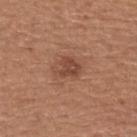<lesion>
<biopsy_status>not biopsied; imaged during a skin examination</biopsy_status>
<image>
  <source>total-body photography crop</source>
  <field_of_view_mm>15</field_of_view_mm>
</image>
<patient>
  <sex>female</sex>
  <age_approx>65</age_approx>
</patient>
<site>arm</site>
<automated_metrics>
  <area_mm2_approx>6.0</area_mm2_approx>
  <shape_asymmetry>0.35</shape_asymmetry>
  <cielab_L>46</cielab_L>
  <cielab_a>22</cielab_a>
  <cielab_b>29</cielab_b>
  <vs_skin_darker_L>9.0</vs_skin_darker_L>
  <vs_skin_contrast_norm>7.0</vs_skin_contrast_norm>
  <border_irregularity_0_10>4.0</border_irregularity_0_10>
  <color_variation_0_10>2.0</color_variation_0_10>
  <peripheral_color_asymmetry>1.0</peripheral_color_asymmetry>
  <lesion_detection_confidence_0_100>100</lesion_detection_confidence_0_100>
</automated_metrics>
<lesion_size>
  <long_diameter_mm_approx>3.5</long_diameter_mm_approx>
</lesion_size>
</lesion>